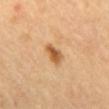Captured during whole-body skin photography for melanoma surveillance; the lesion was not biopsied. The lesion-visualizer software estimated a color-variation rating of about 3.5/10 and peripheral color asymmetry of about 1. The tile uses cross-polarized illumination. A female subject roughly 60 years of age. A roughly 15 mm field-of-view crop from a total-body skin photograph. The lesion is on the mid back.